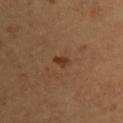follow-up: catalogued during a skin exam; not biopsied
subject: female, aged around 30
acquisition: total-body-photography crop, ~15 mm field of view
tile lighting: cross-polarized illumination
diameter: ≈2 mm
location: the upper back